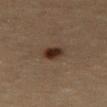Imaged during a routine full-body skin examination; the lesion was not biopsied and no histopathology is available.
Automated image analysis of the tile measured a lesion color around L≈26 a*≈14 b*≈21 in CIELAB and about 11 CIELAB-L* units darker than the surrounding skin. And it measured a border-irregularity index near 1.5/10, a color-variation rating of about 5/10, and peripheral color asymmetry of about 1.5. The software also gave a detector confidence of about 100 out of 100 that the crop contains a lesion.
This image is a 15 mm lesion crop taken from a total-body photograph.
The lesion is on the right thigh.
The recorded lesion diameter is about 3 mm.
The patient is a female about 65 years old.